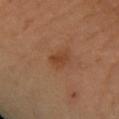Impression: Imaged during a routine full-body skin examination; the lesion was not biopsied and no histopathology is available. Image and clinical context: From the left forearm. A 15 mm close-up tile from a total-body photography series done for melanoma screening. A female subject aged approximately 55.